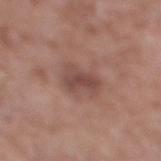biopsy status = imaged on a skin check; not biopsied | patient = male, aged 58 to 62 | diameter = ~3.5 mm (longest diameter) | location = the left lower leg | imaging modality = ~15 mm tile from a whole-body skin photo | TBP lesion metrics = an eccentricity of roughly 0.75; a nevus-likeness score of about 5/100 and lesion-presence confidence of about 100/100 | lighting = white-light.